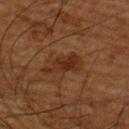The lesion was tiled from a total-body skin photograph and was not biopsied.
The subject is a male aged 63 to 67.
The total-body-photography lesion software estimated a nevus-likeness score of about 5/100 and a detector confidence of about 100 out of 100 that the crop contains a lesion.
Approximately 4.5 mm at its widest.
A 15 mm close-up tile from a total-body photography series done for melanoma screening.
This is a cross-polarized tile.
From the upper back.